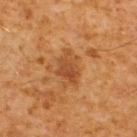workup = imaged on a skin check; not biopsied | subject = male, in their 60s | site = the upper back | acquisition = ~15 mm crop, total-body skin-cancer survey.